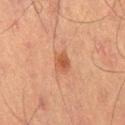Clinical impression:
Part of a total-body skin-imaging series; this lesion was reviewed on a skin check and was not flagged for biopsy.
Acquisition and patient details:
This is a cross-polarized tile. On the left thigh. The recorded lesion diameter is about 2.5 mm. An algorithmic analysis of the crop reported a lesion area of about 4.5 mm² and two-axis asymmetry of about 0.25. It also reported a lesion–skin lightness drop of about 8 and a lesion-to-skin contrast of about 7 (normalized; higher = more distinct). The software also gave a border-irregularity index near 2.5/10, a color-variation rating of about 2.5/10, and peripheral color asymmetry of about 1. Cropped from a whole-body photographic skin survey; the tile spans about 15 mm. A male subject about 65 years old.